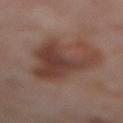The lesion was photographed on a routine skin check and not biopsied; there is no pathology result. A roughly 15 mm field-of-view crop from a total-body skin photograph. The lesion's longest dimension is about 9.5 mm. Automated tile analysis of the lesion measured a border-irregularity rating of about 8/10 and a color-variation rating of about 5/10. And it measured an automated nevus-likeness rating near 20 out of 100. Captured under cross-polarized illumination. The patient is a female in their mid- to late 50s. From the right thigh.